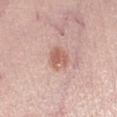The lesion was photographed on a routine skin check and not biopsied; there is no pathology result. Located on the right lower leg. A female patient about 65 years old. A 15 mm crop from a total-body photograph taken for skin-cancer surveillance. Captured under white-light illumination. The total-body-photography lesion software estimated an area of roughly 5.5 mm² and an outline eccentricity of about 0.7 (0 = round, 1 = elongated). The software also gave a border-irregularity rating of about 2/10, internal color variation of about 3 on a 0–10 scale, and peripheral color asymmetry of about 1. The software also gave an automated nevus-likeness rating near 85 out of 100.